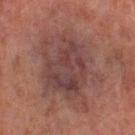Assessment: Recorded during total-body skin imaging; not selected for excision or biopsy. Background: A region of skin cropped from a whole-body photographic capture, roughly 15 mm wide. A female subject aged 58–62. Automated image analysis of the tile measured a nevus-likeness score of about 20/100. Approximately 9.5 mm at its widest. On the leg. Imaged with cross-polarized lighting.